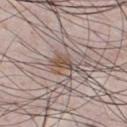follow-up: imaged on a skin check; not biopsied
lighting: white-light illumination
image source: ~15 mm crop, total-body skin-cancer survey
size: ≈3 mm
site: the chest
patient: male, in their mid- to late 30s